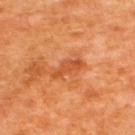Clinical impression: The lesion was tiled from a total-body skin photograph and was not biopsied. Acquisition and patient details: The patient is a male aged 63–67. Located on the back. Automated tile analysis of the lesion measured a lesion color around L≈51 a*≈32 b*≈43 in CIELAB, roughly 9 lightness units darker than nearby skin, and a normalized lesion–skin contrast near 6.5. The analysis additionally found an automated nevus-likeness rating near 0 out of 100 and a lesion-detection confidence of about 100/100. The recorded lesion diameter is about 4 mm. This image is a 15 mm lesion crop taken from a total-body photograph.